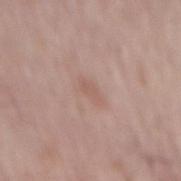Assessment: Recorded during total-body skin imaging; not selected for excision or biopsy. Image and clinical context: On the mid back. A male patient approximately 65 years of age. Captured under white-light illumination. About 2.5 mm across. A 15 mm close-up tile from a total-body photography series done for melanoma screening.